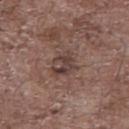Clinical impression: No biopsy was performed on this lesion — it was imaged during a full skin examination and was not determined to be concerning. Clinical summary: The lesion is on the front of the torso. A male subject aged 68–72. Cropped from a whole-body photographic skin survey; the tile spans about 15 mm. Measured at roughly 3.5 mm in maximum diameter.